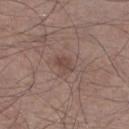The lesion was photographed on a routine skin check and not biopsied; there is no pathology result.
Longest diameter approximately 2.5 mm.
The patient is a male in their mid- to late 60s.
A 15 mm close-up extracted from a 3D total-body photography capture.
The lesion-visualizer software estimated a lesion area of about 4 mm² and two-axis asymmetry of about 0.25. And it measured a mean CIELAB color near L≈45 a*≈17 b*≈22 and roughly 7 lightness units darker than nearby skin. It also reported a classifier nevus-likeness of about 5/100 and a lesion-detection confidence of about 100/100.
Located on the right lower leg.